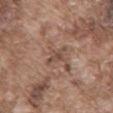biopsy status=imaged on a skin check; not biopsied | image=15 mm crop, total-body photography | patient=male, roughly 75 years of age | site=the mid back.